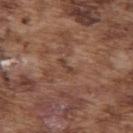<record>
  <biopsy_status>not biopsied; imaged during a skin examination</biopsy_status>
</record>